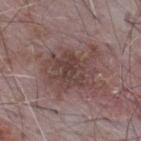notes: no biopsy performed (imaged during a skin exam)
image-analysis metrics: peripheral color asymmetry of about 1.5; an automated nevus-likeness rating near 0 out of 100 and a detector confidence of about 100 out of 100 that the crop contains a lesion
illumination: white-light
anatomic site: the mid back
patient: male, aged around 65
acquisition: ~15 mm tile from a whole-body skin photo